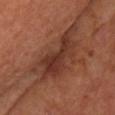The lesion was photographed on a routine skin check and not biopsied; there is no pathology result. The subject is in their mid-50s. From the back. This is a cross-polarized tile. A region of skin cropped from a whole-body photographic capture, roughly 15 mm wide. Longest diameter approximately 7.5 mm. The total-body-photography lesion software estimated an outline eccentricity of about 0.9 (0 = round, 1 = elongated) and a shape-asymmetry score of about 0.45 (0 = symmetric). The software also gave an average lesion color of about L≈33 a*≈23 b*≈27 (CIELAB). It also reported a border-irregularity index near 7/10 and peripheral color asymmetry of about 1.